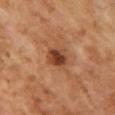Q: Was this lesion biopsied?
A: total-body-photography surveillance lesion; no biopsy
Q: What kind of image is this?
A: ~15 mm crop, total-body skin-cancer survey
Q: What is the anatomic site?
A: the chest
Q: Who is the patient?
A: female, aged 58 to 62
Q: Lesion size?
A: ~2.5 mm (longest diameter)
Q: Automated lesion metrics?
A: an outline eccentricity of about 0.55 (0 = round, 1 = elongated); a mean CIELAB color near L≈36 a*≈21 b*≈30, a lesion–skin lightness drop of about 12, and a normalized border contrast of about 10; a detector confidence of about 100 out of 100 that the crop contains a lesion